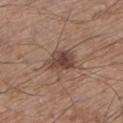<case>
<biopsy_status>not biopsied; imaged during a skin examination</biopsy_status>
<lesion_size>
  <long_diameter_mm_approx>4.0</long_diameter_mm_approx>
</lesion_size>
<patient>
  <sex>male</sex>
  <age_approx>65</age_approx>
</patient>
<site>left lower leg</site>
<automated_metrics>
  <border_irregularity_0_10>2.0</border_irregularity_0_10>
  <color_variation_0_10>4.5</color_variation_0_10>
  <peripheral_color_asymmetry>1.5</peripheral_color_asymmetry>
</automated_metrics>
<image>
  <source>total-body photography crop</source>
  <field_of_view_mm>15</field_of_view_mm>
</image>
</case>